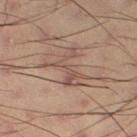Case summary:
- diameter · about 5 mm
- patient · male, in their mid-50s
- anatomic site · the left thigh
- illumination · cross-polarized illumination
- imaging modality · 15 mm crop, total-body photography